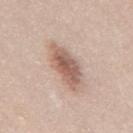  biopsy_status: not biopsied; imaged during a skin examination
  patient:
    sex: male
    age_approx: 45
  lighting: white-light
  image:
    source: total-body photography crop
    field_of_view_mm: 15
  site: back
  lesion_size:
    long_diameter_mm_approx: 5.5